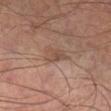workup: imaged on a skin check; not biopsied
site: the right lower leg
patient: male, roughly 60 years of age
imaging modality: total-body-photography crop, ~15 mm field of view
automated metrics: an outline eccentricity of about 0.75 (0 = round, 1 = elongated) and a shape-asymmetry score of about 0.25 (0 = symmetric); a lesion color around L≈47 a*≈17 b*≈26 in CIELAB, a lesion–skin lightness drop of about 7, and a normalized lesion–skin contrast near 5.5; a border-irregularity index near 2.5/10 and radial color variation of about 1; an automated nevus-likeness rating near 0 out of 100 and a detector confidence of about 100 out of 100 that the crop contains a lesion